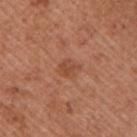Clinical impression: The lesion was photographed on a routine skin check and not biopsied; there is no pathology result. Clinical summary: Located on the right upper arm. A male subject roughly 55 years of age. Approximately 2.5 mm at its widest. A 15 mm crop from a total-body photograph taken for skin-cancer surveillance.